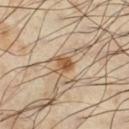Captured under cross-polarized illumination.
From the leg.
A region of skin cropped from a whole-body photographic capture, roughly 15 mm wide.
The total-body-photography lesion software estimated a nevus-likeness score of about 85/100.
A male subject, about 40 years old.